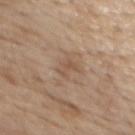The tile uses white-light illumination. A male subject, aged approximately 70. Cropped from a total-body skin-imaging series; the visible field is about 15 mm. Longest diameter approximately 3 mm. The lesion is located on the chest.